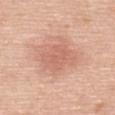follow-up: catalogued during a skin exam; not biopsied
body site: the upper back
lesion diameter: about 4.5 mm
illumination: white-light illumination
subject: male, aged 68–72
imaging modality: ~15 mm tile from a whole-body skin photo
automated metrics: an area of roughly 11 mm² and an outline eccentricity of about 0.65 (0 = round, 1 = elongated)